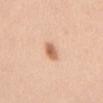Clinical summary: The patient is a female approximately 30 years of age. An algorithmic analysis of the crop reported an area of roughly 4 mm², an outline eccentricity of about 0.8 (0 = round, 1 = elongated), and a shape-asymmetry score of about 0.25 (0 = symmetric). The analysis additionally found a border-irregularity index near 2.5/10, a within-lesion color-variation index near 2.5/10, and radial color variation of about 0.5. The software also gave a classifier nevus-likeness of about 100/100. Captured under white-light illumination. The lesion is located on the front of the torso. A region of skin cropped from a whole-body photographic capture, roughly 15 mm wide.